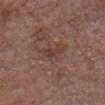biopsy_status: not biopsied; imaged during a skin examination
automated_metrics:
  area_mm2_approx: 3.0
  shape_asymmetry: 0.4
patient:
  sex: male
  age_approx: 80
site: head or neck
lighting: white-light
image:
  source: total-body photography crop
  field_of_view_mm: 15
lesion_size:
  long_diameter_mm_approx: 3.0